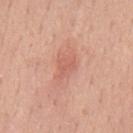notes: catalogued during a skin exam; not biopsied
subject: male, approximately 50 years of age
anatomic site: the back
imaging modality: ~15 mm tile from a whole-body skin photo
lesion diameter: about 3 mm
lighting: white-light illumination
automated metrics: a lesion area of about 4 mm², a shape eccentricity near 0.65, and a shape-asymmetry score of about 0.5 (0 = symmetric); a mean CIELAB color near L≈61 a*≈27 b*≈30, a lesion–skin lightness drop of about 7, and a normalized lesion–skin contrast near 4.5; internal color variation of about 1.5 on a 0–10 scale and peripheral color asymmetry of about 0.5